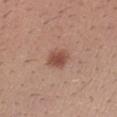biopsy_status: not biopsied; imaged during a skin examination